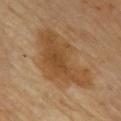Q: Is there a histopathology result?
A: total-body-photography surveillance lesion; no biopsy
Q: Who is the patient?
A: male, approximately 65 years of age
Q: How was this image acquired?
A: 15 mm crop, total-body photography
Q: What is the anatomic site?
A: the chest